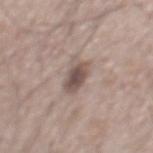{
  "biopsy_status": "not biopsied; imaged during a skin examination",
  "patient": {
    "sex": "male",
    "age_approx": 65
  },
  "lesion_size": {
    "long_diameter_mm_approx": 4.0
  },
  "site": "chest",
  "automated_metrics": {
    "area_mm2_approx": 7.5,
    "eccentricity": 0.85,
    "shape_asymmetry": 0.35,
    "vs_skin_darker_L": 12.0,
    "vs_skin_contrast_norm": 8.5,
    "border_irregularity_0_10": 3.5,
    "color_variation_0_10": 4.0,
    "peripheral_color_asymmetry": 1.0,
    "lesion_detection_confidence_0_100": 100
  },
  "image": {
    "source": "total-body photography crop",
    "field_of_view_mm": 15
  }
}